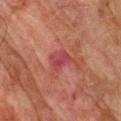Q: Was this lesion biopsied?
A: total-body-photography surveillance lesion; no biopsy
Q: Where on the body is the lesion?
A: the upper back
Q: What kind of image is this?
A: ~15 mm crop, total-body skin-cancer survey
Q: What is the lesion's diameter?
A: ≈3 mm
Q: Patient demographics?
A: male, about 75 years old